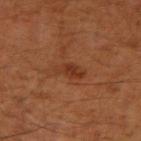Imaged during a routine full-body skin examination; the lesion was not biopsied and no histopathology is available.